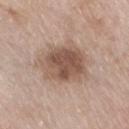Notes:
- notes: catalogued during a skin exam; not biopsied